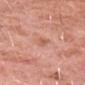Imaged during a routine full-body skin examination; the lesion was not biopsied and no histopathology is available. A male subject, aged around 80. The lesion is located on the head or neck. This image is a 15 mm lesion crop taken from a total-body photograph. Captured under white-light illumination.